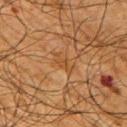Q: Is there a histopathology result?
A: catalogued during a skin exam; not biopsied
Q: What lighting was used for the tile?
A: cross-polarized illumination
Q: Lesion location?
A: the right upper arm
Q: What did automated image analysis measure?
A: a footprint of about 2.5 mm², a shape eccentricity near 0.85, and two-axis asymmetry of about 0.4; a mean CIELAB color near L≈39 a*≈20 b*≈34, roughly 5 lightness units darker than nearby skin, and a normalized border contrast of about 5.5; a border-irregularity rating of about 4.5/10, internal color variation of about 0 on a 0–10 scale, and radial color variation of about 0
Q: What is the imaging modality?
A: ~15 mm crop, total-body skin-cancer survey
Q: Patient demographics?
A: male, in their mid- to late 60s